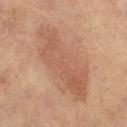workup: imaged on a skin check; not biopsied
patient: female, about 60 years old
location: the left thigh
diameter: ≈9.5 mm
illumination: cross-polarized
image source: total-body-photography crop, ~15 mm field of view